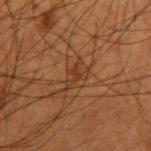Imaged during a routine full-body skin examination; the lesion was not biopsied and no histopathology is available. The subject is a male aged approximately 50. The lesion is located on the right upper arm. The total-body-photography lesion software estimated an area of roughly 4.5 mm² and a symmetry-axis asymmetry near 0.55. It also reported border irregularity of about 7 on a 0–10 scale and a peripheral color-asymmetry measure near 0.5. It also reported a classifier nevus-likeness of about 0/100 and a lesion-detection confidence of about 90/100. This image is a 15 mm lesion crop taken from a total-body photograph. The tile uses cross-polarized illumination. Longest diameter approximately 3 mm.